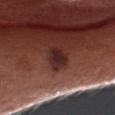Part of a total-body skin-imaging series; this lesion was reviewed on a skin check and was not flagged for biopsy.
A male subject aged 63 to 67.
A region of skin cropped from a whole-body photographic capture, roughly 15 mm wide.
On the head or neck.